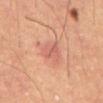biopsy status = total-body-photography surveillance lesion; no biopsy | image source = total-body-photography crop, ~15 mm field of view | patient = male, in their mid-50s | lighting = cross-polarized illumination | size = ≈3 mm | anatomic site = the abdomen.